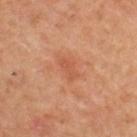{
  "biopsy_status": "not biopsied; imaged during a skin examination",
  "lesion_size": {
    "long_diameter_mm_approx": 3.0
  },
  "site": "upper back",
  "automated_metrics": {
    "area_mm2_approx": 3.5,
    "shape_asymmetry": 0.3,
    "cielab_L": 53,
    "cielab_a": 27,
    "cielab_b": 35,
    "vs_skin_darker_L": 7.0,
    "vs_skin_contrast_norm": 5.0,
    "lesion_detection_confidence_0_100": 100
  },
  "patient": {
    "sex": "male",
    "age_approx": 55
  },
  "image": {
    "source": "total-body photography crop",
    "field_of_view_mm": 15
  }
}